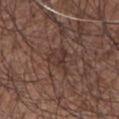workup: catalogued during a skin exam; not biopsied | lighting: white-light illumination | acquisition: ~15 mm crop, total-body skin-cancer survey | lesion size: about 3.5 mm | subject: male, about 60 years old | location: the right upper arm.